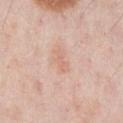follow-up: imaged on a skin check; not biopsied | diameter: ~3 mm (longest diameter) | illumination: white-light illumination | automated metrics: an area of roughly 3.5 mm², an outline eccentricity of about 0.9 (0 = round, 1 = elongated), and a symmetry-axis asymmetry near 0.45 | site: the chest | patient: male, aged around 60 | image source: ~15 mm crop, total-body skin-cancer survey.